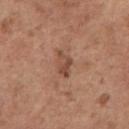The lesion was photographed on a routine skin check and not biopsied; there is no pathology result. Approximately 3 mm at its widest. A female subject about 65 years old. Captured under white-light illumination. The lesion is on the right upper arm. Cropped from a whole-body photographic skin survey; the tile spans about 15 mm.